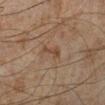No biopsy was performed on this lesion — it was imaged during a full skin examination and was not determined to be concerning. A lesion tile, about 15 mm wide, cut from a 3D total-body photograph. Located on the left lower leg. The patient is a male approximately 45 years of age.